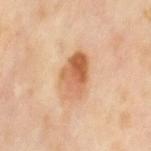The lesion was photographed on a routine skin check and not biopsied; there is no pathology result. The subject is a female aged 48 to 52. A 15 mm close-up tile from a total-body photography series done for melanoma screening. The lesion is on the left thigh.